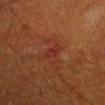Impression:
Part of a total-body skin-imaging series; this lesion was reviewed on a skin check and was not flagged for biopsy.
Context:
This image is a 15 mm lesion crop taken from a total-body photograph. A male patient, aged 58 to 62. From the right upper arm.